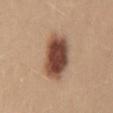follow-up: no biopsy performed (imaged during a skin exam) | subject: female, aged 23 to 27 | imaging modality: ~15 mm tile from a whole-body skin photo | lesion diameter: ≈6 mm | lighting: white-light | body site: the lower back.